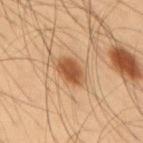notes=catalogued during a skin exam; not biopsied
image-analysis metrics=a within-lesion color-variation index near 3.5/10 and peripheral color asymmetry of about 1
illumination=cross-polarized
size=≈4 mm
anatomic site=the mid back
subject=male, aged 53 to 57
image=~15 mm crop, total-body skin-cancer survey